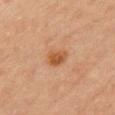| field | value |
|---|---|
| notes | no biopsy performed (imaged during a skin exam) |
| acquisition | ~15 mm crop, total-body skin-cancer survey |
| location | the front of the torso |
| subject | female, roughly 65 years of age |